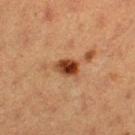Assessment: Recorded during total-body skin imaging; not selected for excision or biopsy.